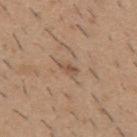Clinical impression:
No biopsy was performed on this lesion — it was imaged during a full skin examination and was not determined to be concerning.
Clinical summary:
Automated image analysis of the tile measured a shape eccentricity near 0.85. The software also gave a border-irregularity rating of about 2.5/10 and a peripheral color-asymmetry measure near 1. And it measured a classifier nevus-likeness of about 0/100 and lesion-presence confidence of about 95/100. A roughly 15 mm field-of-view crop from a total-body skin photograph. This is a white-light tile. The lesion's longest dimension is about 2.5 mm. A male subject aged around 40. From the back.